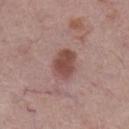Recorded during total-body skin imaging; not selected for excision or biopsy.
Cropped from a total-body skin-imaging series; the visible field is about 15 mm.
Located on the left lower leg.
A female patient in their mid- to late 60s.